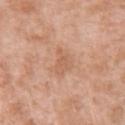The lesion was tiled from a total-body skin photograph and was not biopsied.
From the right upper arm.
A roughly 15 mm field-of-view crop from a total-body skin photograph.
The total-body-photography lesion software estimated a footprint of about 4.5 mm², an outline eccentricity of about 0.45 (0 = round, 1 = elongated), and a symmetry-axis asymmetry near 0.4. The analysis additionally found a within-lesion color-variation index near 1/10.
The tile uses white-light illumination.
Measured at roughly 3 mm in maximum diameter.
The patient is a female aged 38–42.